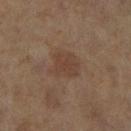No biopsy was performed on this lesion — it was imaged during a full skin examination and was not determined to be concerning. The lesion is on the leg. The recorded lesion diameter is about 3.5 mm. An algorithmic analysis of the crop reported an area of roughly 8.5 mm² and an eccentricity of roughly 0.55. It also reported a mean CIELAB color near L≈35 a*≈14 b*≈23, a lesion–skin lightness drop of about 5, and a lesion-to-skin contrast of about 5.5 (normalized; higher = more distinct). And it measured an automated nevus-likeness rating near 5 out of 100 and a detector confidence of about 100 out of 100 that the crop contains a lesion. Cropped from a total-body skin-imaging series; the visible field is about 15 mm. A female subject aged approximately 60. The tile uses cross-polarized illumination.